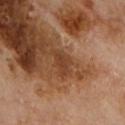Impression:
Imaged during a routine full-body skin examination; the lesion was not biopsied and no histopathology is available.
Clinical summary:
The patient is a male aged approximately 70. Automated image analysis of the tile measured an area of roughly 5 mm², an outline eccentricity of about 0.8 (0 = round, 1 = elongated), and a shape-asymmetry score of about 0.45 (0 = symmetric). And it measured a border-irregularity rating of about 4.5/10, a color-variation rating of about 2/10, and peripheral color asymmetry of about 0.5. It also reported a classifier nevus-likeness of about 0/100. A 15 mm close-up tile from a total-body photography series done for melanoma screening. The lesion is on the front of the torso. Approximately 3.5 mm at its widest. Imaged with cross-polarized lighting.